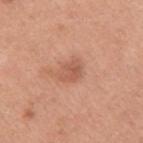follow-up = catalogued during a skin exam; not biopsied | body site = the right upper arm | tile lighting = white-light illumination | image source = 15 mm crop, total-body photography | subject = male, aged approximately 30.